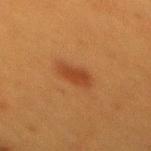Part of a total-body skin-imaging series; this lesion was reviewed on a skin check and was not flagged for biopsy. The patient is a female about 40 years old. A lesion tile, about 15 mm wide, cut from a 3D total-body photograph. From the mid back.